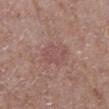This lesion was catalogued during total-body skin photography and was not selected for biopsy. Imaged with white-light lighting. A close-up tile cropped from a whole-body skin photograph, about 15 mm across. The recorded lesion diameter is about 4 mm. On the right lower leg. A male subject about 65 years old. An algorithmic analysis of the crop reported a lesion color around L≈50 a*≈21 b*≈21 in CIELAB and a lesion–skin lightness drop of about 6. The software also gave peripheral color asymmetry of about 0.5. And it measured an automated nevus-likeness rating near 0 out of 100 and a lesion-detection confidence of about 100/100.